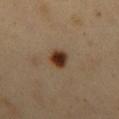Impression: The lesion was tiled from a total-body skin photograph and was not biopsied. Background: A lesion tile, about 15 mm wide, cut from a 3D total-body photograph. The patient is a male in their 50s. From the mid back. Automated image analysis of the tile measured a nevus-likeness score of about 100/100 and a detector confidence of about 100 out of 100 that the crop contains a lesion.